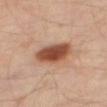No biopsy was performed on this lesion — it was imaged during a full skin examination and was not determined to be concerning. A roughly 15 mm field-of-view crop from a total-body skin photograph. Approximately 5 mm at its widest. The lesion is on the right thigh. The subject is a male in their mid-50s.